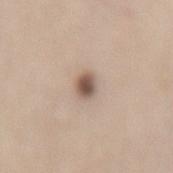Part of a total-body skin-imaging series; this lesion was reviewed on a skin check and was not flagged for biopsy. Approximately 2.5 mm at its widest. A 15 mm crop from a total-body photograph taken for skin-cancer surveillance. The patient is a female about 50 years old. The lesion is located on the mid back. Captured under white-light illumination.